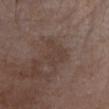<tbp_lesion>
<biopsy_status>not biopsied; imaged during a skin examination</biopsy_status>
<lesion_size>
  <long_diameter_mm_approx>4.0</long_diameter_mm_approx>
</lesion_size>
<lighting>white-light</lighting>
<patient>
  <sex>female</sex>
  <age_approx>80</age_approx>
</patient>
<site>head or neck</site>
<image>
  <source>total-body photography crop</source>
  <field_of_view_mm>15</field_of_view_mm>
</image>
</tbp_lesion>